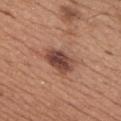notes: no biopsy performed (imaged during a skin exam)
anatomic site: the front of the torso
acquisition: ~15 mm crop, total-body skin-cancer survey
diameter: ≈4 mm
patient: male, roughly 65 years of age